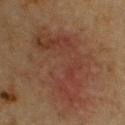Q: Is there a histopathology result?
A: imaged on a skin check; not biopsied
Q: What are the patient's age and sex?
A: male, aged approximately 75
Q: What is the lesion's diameter?
A: about 10 mm
Q: What is the imaging modality?
A: ~15 mm crop, total-body skin-cancer survey
Q: What did automated image analysis measure?
A: a lesion color around L≈30 a*≈18 b*≈22 in CIELAB, about 5 CIELAB-L* units darker than the surrounding skin, and a normalized lesion–skin contrast near 5.5; a classifier nevus-likeness of about 0/100
Q: Where on the body is the lesion?
A: the chest
Q: How was the tile lit?
A: cross-polarized